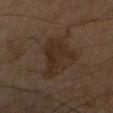This lesion was catalogued during total-body skin photography and was not selected for biopsy. The subject is a male aged around 65. Measured at roughly 5.5 mm in maximum diameter. The total-body-photography lesion software estimated a shape eccentricity near 0.65 and a symmetry-axis asymmetry near 0.5. The software also gave an average lesion color of about L≈26 a*≈13 b*≈23 (CIELAB), about 6 CIELAB-L* units darker than the surrounding skin, and a lesion-to-skin contrast of about 7 (normalized; higher = more distinct). The lesion is on the arm. A 15 mm close-up extracted from a 3D total-body photography capture.